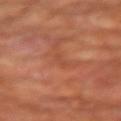Impression: Captured during whole-body skin photography for melanoma surveillance; the lesion was not biopsied. Clinical summary: A male subject about 65 years old. Located on the right upper arm. A region of skin cropped from a whole-body photographic capture, roughly 15 mm wide.